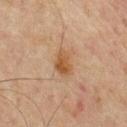Notes:
- biopsy status — imaged on a skin check; not biopsied
- automated lesion analysis — an average lesion color of about L≈42 a*≈16 b*≈31 (CIELAB), a lesion–skin lightness drop of about 8, and a normalized border contrast of about 8; a border-irregularity rating of about 2.5/10, internal color variation of about 3.5 on a 0–10 scale, and a peripheral color-asymmetry measure near 1.5; an automated nevus-likeness rating near 35 out of 100 and lesion-presence confidence of about 100/100
- lesion size — ~3 mm (longest diameter)
- patient — male, aged approximately 65
- anatomic site — the mid back
- imaging modality — 15 mm crop, total-body photography
- illumination — cross-polarized illumination